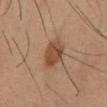Impression: No biopsy was performed on this lesion — it was imaged during a full skin examination and was not determined to be concerning. Clinical summary: A male subject aged approximately 40. The lesion is on the mid back. Approximately 4 mm at its widest. Automated image analysis of the tile measured a lesion color around L≈46 a*≈20 b*≈31 in CIELAB, about 10 CIELAB-L* units darker than the surrounding skin, and a normalized lesion–skin contrast near 8. The software also gave a classifier nevus-likeness of about 100/100 and a lesion-detection confidence of about 100/100. A region of skin cropped from a whole-body photographic capture, roughly 15 mm wide. Captured under cross-polarized illumination.